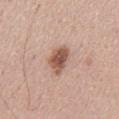<lesion>
  <biopsy_status>not biopsied; imaged during a skin examination</biopsy_status>
  <lesion_size>
    <long_diameter_mm_approx>4.0</long_diameter_mm_approx>
  </lesion_size>
  <site>abdomen</site>
  <lighting>white-light</lighting>
  <patient>
    <sex>male</sex>
    <age_approx>55</age_approx>
  </patient>
  <automated_metrics>
    <cielab_L>54</cielab_L>
    <cielab_a>21</cielab_a>
    <cielab_b>27</cielab_b>
    <vs_skin_darker_L>14.0</vs_skin_darker_L>
    <vs_skin_contrast_norm>9.5</vs_skin_contrast_norm>
  </automated_metrics>
  <image>
    <source>total-body photography crop</source>
    <field_of_view_mm>15</field_of_view_mm>
  </image>
</lesion>